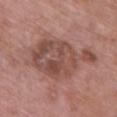The lesion was photographed on a routine skin check and not biopsied; there is no pathology result. Longest diameter approximately 9 mm. The subject is a female roughly 65 years of age. This image is a 15 mm lesion crop taken from a total-body photograph. From the upper back. Automated image analysis of the tile measured an area of roughly 34 mm² and a shape eccentricity near 0.8. The analysis additionally found a border-irregularity rating of about 4.5/10 and radial color variation of about 1.5.